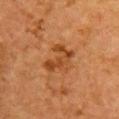anatomic site: the chest
imaging modality: ~15 mm crop, total-body skin-cancer survey
patient: female, approximately 50 years of age